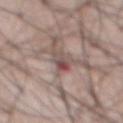No biopsy was performed on this lesion — it was imaged during a full skin examination and was not determined to be concerning. From the abdomen. A lesion tile, about 15 mm wide, cut from a 3D total-body photograph. A male patient, aged approximately 60.